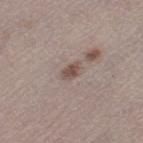| field | value |
|---|---|
| notes | imaged on a skin check; not biopsied |
| size | about 2.5 mm |
| TBP lesion metrics | a lesion area of about 3.5 mm² and a symmetry-axis asymmetry near 0.2; border irregularity of about 2 on a 0–10 scale and a within-lesion color-variation index near 2/10; a lesion-detection confidence of about 100/100 |
| illumination | white-light illumination |
| image | 15 mm crop, total-body photography |
| location | the left thigh |
| subject | female, aged 48–52 |